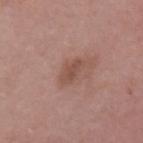A 15 mm crop from a total-body photograph taken for skin-cancer surveillance. The subject is a female in their mid- to late 40s. On the left upper arm.